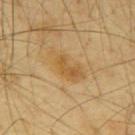Assessment: No biopsy was performed on this lesion — it was imaged during a full skin examination and was not determined to be concerning. Context: Imaged with cross-polarized lighting. From the back. The recorded lesion diameter is about 5 mm. The lesion-visualizer software estimated a color-variation rating of about 4.5/10 and radial color variation of about 1.5. Cropped from a whole-body photographic skin survey; the tile spans about 15 mm. A male patient aged 63 to 67.